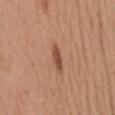The lesion was photographed on a routine skin check and not biopsied; there is no pathology result. A close-up tile cropped from a whole-body skin photograph, about 15 mm across. The total-body-photography lesion software estimated an area of roughly 3 mm², an eccentricity of roughly 0.95, and a symmetry-axis asymmetry near 0.3. And it measured an average lesion color of about L≈49 a*≈23 b*≈31 (CIELAB), a lesion–skin lightness drop of about 12, and a lesion-to-skin contrast of about 8.5 (normalized; higher = more distinct). The software also gave border irregularity of about 3.5 on a 0–10 scale, a color-variation rating of about 0/10, and radial color variation of about 0. A female patient about 40 years old. Located on the back.